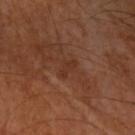Q: Where on the body is the lesion?
A: the left upper arm
Q: How was this image acquired?
A: ~15 mm crop, total-body skin-cancer survey
Q: What are the patient's age and sex?
A: male, aged 58 to 62